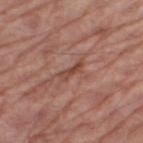• biopsy status — imaged on a skin check; not biopsied
• image — ~15 mm tile from a whole-body skin photo
• subject — male, approximately 70 years of age
• lesion diameter — about 3 mm
• site — the right thigh